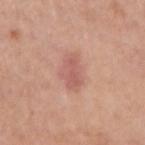Q: Is there a histopathology result?
A: imaged on a skin check; not biopsied
Q: What is the imaging modality?
A: total-body-photography crop, ~15 mm field of view
Q: Where on the body is the lesion?
A: the right upper arm
Q: How large is the lesion?
A: ≈4.5 mm
Q: Who is the patient?
A: male, aged 73–77
Q: What lighting was used for the tile?
A: white-light illumination